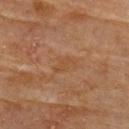biopsy status=imaged on a skin check; not biopsied | subject=female, aged 78–82 | image=~15 mm tile from a whole-body skin photo | body site=the back.